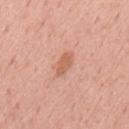Imaged during a routine full-body skin examination; the lesion was not biopsied and no histopathology is available. Cropped from a total-body skin-imaging series; the visible field is about 15 mm. Imaged with white-light lighting. A male patient, roughly 55 years of age. The lesion is located on the mid back. The recorded lesion diameter is about 3 mm.